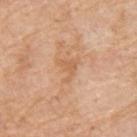{
  "biopsy_status": "not biopsied; imaged during a skin examination",
  "lesion_size": {
    "long_diameter_mm_approx": 3.0
  },
  "patient": {
    "sex": "male",
    "age_approx": 60
  },
  "automated_metrics": {
    "eccentricity": 0.6,
    "nevus_likeness_0_100": 0,
    "lesion_detection_confidence_0_100": 100
  },
  "lighting": "white-light",
  "site": "upper back",
  "image": {
    "source": "total-body photography crop",
    "field_of_view_mm": 15
  }
}